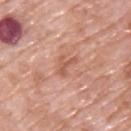Captured during whole-body skin photography for melanoma surveillance; the lesion was not biopsied.
The lesion is located on the upper back.
The recorded lesion diameter is about 3 mm.
Imaged with white-light lighting.
A male subject in their 70s.
Cropped from a whole-body photographic skin survey; the tile spans about 15 mm.